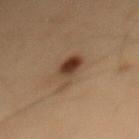• TBP lesion metrics · roughly 10 lightness units darker than nearby skin
• image source · ~15 mm crop, total-body skin-cancer survey
• patient · male, about 55 years old
• site · the mid back
• size · ≈5 mm